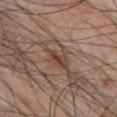– patient: male, aged approximately 70
– image: ~15 mm tile from a whole-body skin photo
– tile lighting: white-light illumination
– size: ~4 mm (longest diameter)
– location: the front of the torso